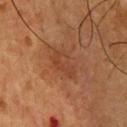Impression: Part of a total-body skin-imaging series; this lesion was reviewed on a skin check and was not flagged for biopsy. Clinical summary: Automated image analysis of the tile measured a lesion area of about 6.5 mm² and an outline eccentricity of about 0.85 (0 = round, 1 = elongated). The software also gave a border-irregularity rating of about 3.5/10, a color-variation rating of about 3.5/10, and peripheral color asymmetry of about 1. A 15 mm crop from a total-body photograph taken for skin-cancer surveillance. Longest diameter approximately 4 mm. A male subject aged approximately 55. Captured under cross-polarized illumination. On the chest.